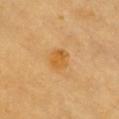Notes:
* follow-up — total-body-photography surveillance lesion; no biopsy
* image — 15 mm crop, total-body photography
* patient — male, roughly 60 years of age
* site — the chest